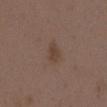Q: Was this lesion biopsied?
A: total-body-photography surveillance lesion; no biopsy
Q: How was this image acquired?
A: ~15 mm crop, total-body skin-cancer survey
Q: What is the lesion's diameter?
A: about 2.5 mm
Q: What are the patient's age and sex?
A: female, aged 33 to 37
Q: Lesion location?
A: the upper back
Q: Automated lesion metrics?
A: a lesion area of about 4 mm² and a symmetry-axis asymmetry near 0.2; border irregularity of about 2 on a 0–10 scale, internal color variation of about 1.5 on a 0–10 scale, and peripheral color asymmetry of about 0.5
Q: Illumination type?
A: white-light illumination